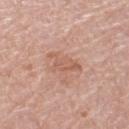The lesion was photographed on a routine skin check and not biopsied; there is no pathology result. Automated tile analysis of the lesion measured a footprint of about 6.5 mm², an eccentricity of roughly 0.8, and a symmetry-axis asymmetry near 0.5. The analysis additionally found a border-irregularity index near 6/10 and a peripheral color-asymmetry measure near 1. The analysis additionally found a nevus-likeness score of about 0/100. A female subject about 70 years old. From the left thigh. A region of skin cropped from a whole-body photographic capture, roughly 15 mm wide.